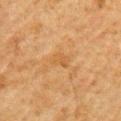Captured during whole-body skin photography for melanoma surveillance; the lesion was not biopsied. The total-body-photography lesion software estimated a footprint of about 3.5 mm² and two-axis asymmetry of about 0.3. The software also gave a color-variation rating of about 1.5/10. The lesion is on the left upper arm. A male patient aged 78–82. Approximately 2.5 mm at its widest. Captured under cross-polarized illumination. A region of skin cropped from a whole-body photographic capture, roughly 15 mm wide.